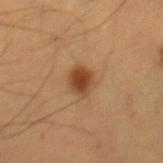Captured during whole-body skin photography for melanoma surveillance; the lesion was not biopsied. The lesion is located on the right thigh. The lesion-visualizer software estimated a lesion color around L≈44 a*≈21 b*≈35 in CIELAB. It also reported a border-irregularity rating of about 1.5/10 and a peripheral color-asymmetry measure near 1. A male patient aged 53 to 57. Imaged with cross-polarized lighting. Longest diameter approximately 3 mm. A 15 mm close-up extracted from a 3D total-body photography capture.